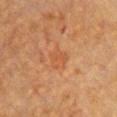notes: total-body-photography surveillance lesion; no biopsy | location: the chest | diameter: ≈2.5 mm | image: ~15 mm tile from a whole-body skin photo | subject: female, aged approximately 55 | automated metrics: a lesion area of about 5 mm², an eccentricity of roughly 0.5, and two-axis asymmetry of about 0.3; an average lesion color of about L≈44 a*≈22 b*≈33 (CIELAB), roughly 5 lightness units darker than nearby skin, and a lesion-to-skin contrast of about 4.5 (normalized; higher = more distinct); an automated nevus-likeness rating near 5 out of 100 and a detector confidence of about 100 out of 100 that the crop contains a lesion.